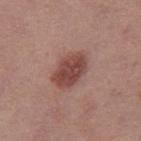Notes:
- site — the right thigh
- automated lesion analysis — a lesion color around L≈45 a*≈24 b*≈24 in CIELAB and roughly 12 lightness units darker than nearby skin; an automated nevus-likeness rating near 95 out of 100 and a lesion-detection confidence of about 100/100
- patient — female, approximately 40 years of age
- diameter — about 4.5 mm
- image — 15 mm crop, total-body photography